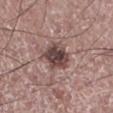{"biopsy_status": "not biopsied; imaged during a skin examination", "lighting": "white-light", "image": {"source": "total-body photography crop", "field_of_view_mm": 15}, "automated_metrics": {"vs_skin_darker_L": 15.0, "vs_skin_contrast_norm": 11.0, "border_irregularity_0_10": 2.5, "color_variation_0_10": 5.5, "lesion_detection_confidence_0_100": 100}, "lesion_size": {"long_diameter_mm_approx": 4.0}, "patient": {"sex": "male", "age_approx": 65}, "site": "leg"}